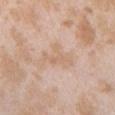workup=imaged on a skin check; not biopsied | diameter=~4.5 mm (longest diameter) | patient=female, aged 23 to 27 | tile lighting=white-light illumination | body site=the chest | image source=total-body-photography crop, ~15 mm field of view.